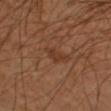Recorded during total-body skin imaging; not selected for excision or biopsy.
Located on the left upper arm.
A male patient, aged approximately 50.
Imaged with cross-polarized lighting.
About 2.5 mm across.
Cropped from a whole-body photographic skin survey; the tile spans about 15 mm.
The lesion-visualizer software estimated a lesion area of about 3 mm² and two-axis asymmetry of about 0.35. It also reported a nevus-likeness score of about 15/100 and a lesion-detection confidence of about 100/100.